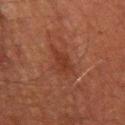Captured under cross-polarized illumination. About 3.5 mm across. The subject is a male aged 43–47. Located on the arm. A 15 mm crop from a total-body photograph taken for skin-cancer surveillance.